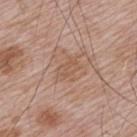Impression:
The lesion was tiled from a total-body skin photograph and was not biopsied.
Clinical summary:
A lesion tile, about 15 mm wide, cut from a 3D total-body photograph. A male patient, approximately 65 years of age. On the upper back.